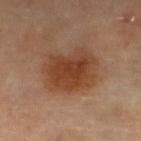The lesion was tiled from a total-body skin photograph and was not biopsied.